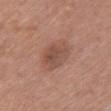Imaged during a routine full-body skin examination; the lesion was not biopsied and no histopathology is available. From the chest. A female subject, aged around 50. About 4 mm across. This is a white-light tile. A lesion tile, about 15 mm wide, cut from a 3D total-body photograph.